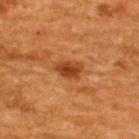Clinical impression: Part of a total-body skin-imaging series; this lesion was reviewed on a skin check and was not flagged for biopsy. Context: Located on the upper back. A lesion tile, about 15 mm wide, cut from a 3D total-body photograph. The subject is a male approximately 60 years of age.